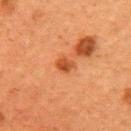This is a cross-polarized tile.
Cropped from a total-body skin-imaging series; the visible field is about 15 mm.
The lesion's longest dimension is about 2.5 mm.
Located on the right upper arm.
A female patient aged around 60.